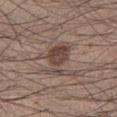notes: no biopsy performed (imaged during a skin exam)